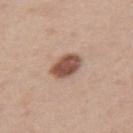The lesion was tiled from a total-body skin photograph and was not biopsied.
About 3.5 mm across.
A roughly 15 mm field-of-view crop from a total-body skin photograph.
The tile uses white-light illumination.
Located on the arm.
A male patient aged approximately 45.
The total-body-photography lesion software estimated a lesion area of about 8 mm², an eccentricity of roughly 0.6, and a symmetry-axis asymmetry near 0.1.